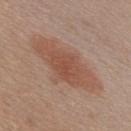Imaged during a routine full-body skin examination; the lesion was not biopsied and no histopathology is available. About 9.5 mm across. The patient is a male aged 43–47. The total-body-photography lesion software estimated an average lesion color of about L≈52 a*≈21 b*≈28 (CIELAB), about 8 CIELAB-L* units darker than the surrounding skin, and a normalized lesion–skin contrast near 6.5. The software also gave a border-irregularity rating of about 4.5/10. And it measured a nevus-likeness score of about 95/100 and lesion-presence confidence of about 100/100. The lesion is located on the front of the torso. Cropped from a total-body skin-imaging series; the visible field is about 15 mm. Imaged with white-light lighting.